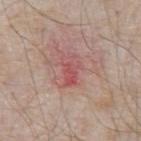<tbp_lesion>
  <biopsy_status>not biopsied; imaged during a skin examination</biopsy_status>
  <image>
    <source>total-body photography crop</source>
    <field_of_view_mm>15</field_of_view_mm>
  </image>
  <patient>
    <sex>male</sex>
    <age_approx>65</age_approx>
  </patient>
  <site>abdomen</site>
</tbp_lesion>